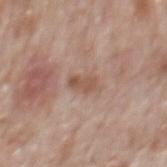| key | value |
|---|---|
| acquisition | 15 mm crop, total-body photography |
| image-analysis metrics | a mean CIELAB color near L≈53 a*≈19 b*≈27, about 9 CIELAB-L* units darker than the surrounding skin, and a normalized lesion–skin contrast near 6.5; a border-irregularity index near 3/10, internal color variation of about 2 on a 0–10 scale, and a peripheral color-asymmetry measure near 0.5 |
| patient | male, aged around 70 |
| anatomic site | the mid back |